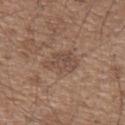{
  "biopsy_status": "not biopsied; imaged during a skin examination",
  "lesion_size": {
    "long_diameter_mm_approx": 4.0
  },
  "patient": {
    "sex": "male",
    "age_approx": 50
  },
  "site": "left upper arm",
  "image": {
    "source": "total-body photography crop",
    "field_of_view_mm": 15
  }
}